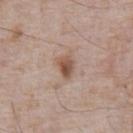workup: total-body-photography surveillance lesion; no biopsy | acquisition: ~15 mm tile from a whole-body skin photo | illumination: white-light illumination | patient: male, aged 68–72 | automated metrics: an eccentricity of roughly 0.7; an automated nevus-likeness rating near 95 out of 100 and a detector confidence of about 100 out of 100 that the crop contains a lesion | site: the front of the torso.